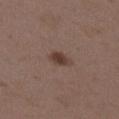Part of a total-body skin-imaging series; this lesion was reviewed on a skin check and was not flagged for biopsy. A female patient aged approximately 35. A lesion tile, about 15 mm wide, cut from a 3D total-body photograph. The lesion is on the upper back.